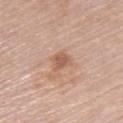Assessment: Part of a total-body skin-imaging series; this lesion was reviewed on a skin check and was not flagged for biopsy. Image and clinical context: Measured at roughly 2.5 mm in maximum diameter. Imaged with white-light lighting. A female patient, about 65 years old. The lesion is on the upper back. This image is a 15 mm lesion crop taken from a total-body photograph.